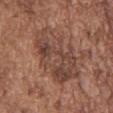Assessment:
Captured during whole-body skin photography for melanoma surveillance; the lesion was not biopsied.
Acquisition and patient details:
Automated tile analysis of the lesion measured an eccentricity of roughly 0.85 and a symmetry-axis asymmetry near 0.25. The software also gave an average lesion color of about L≈44 a*≈20 b*≈26 (CIELAB) and a lesion-to-skin contrast of about 7 (normalized; higher = more distinct). It also reported an automated nevus-likeness rating near 0 out of 100. Imaged with white-light lighting. Located on the chest. A male patient, in their mid-70s. A 15 mm close-up extracted from a 3D total-body photography capture. Approximately 9 mm at its widest.